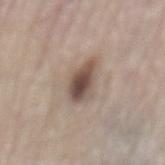Notes:
- follow-up · catalogued during a skin exam; not biopsied
- lesion diameter · ≈4 mm
- acquisition · ~15 mm crop, total-body skin-cancer survey
- subject · male, roughly 80 years of age
- location · the back
- tile lighting · white-light illumination